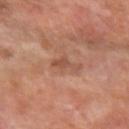Assessment:
The lesion was photographed on a routine skin check and not biopsied; there is no pathology result.
Acquisition and patient details:
The total-body-photography lesion software estimated a footprint of about 3 mm², a shape eccentricity near 0.8, and a shape-asymmetry score of about 0.6 (0 = symmetric). It also reported a mean CIELAB color near L≈48 a*≈22 b*≈29, a lesion–skin lightness drop of about 8, and a lesion-to-skin contrast of about 6 (normalized; higher = more distinct). A male subject, aged approximately 60. The lesion is located on the arm. Imaged with cross-polarized lighting. A region of skin cropped from a whole-body photographic capture, roughly 15 mm wide. The lesion's longest dimension is about 3 mm.